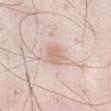Findings:
* notes · total-body-photography surveillance lesion; no biopsy